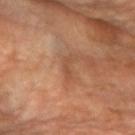The lesion was photographed on a routine skin check and not biopsied; there is no pathology result. A female patient, approximately 70 years of age. The lesion is located on the right forearm. Cropped from a whole-body photographic skin survey; the tile spans about 15 mm.